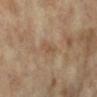follow-up = total-body-photography surveillance lesion; no biopsy
imaging modality = ~15 mm tile from a whole-body skin photo
patient = female, in their mid- to late 70s
diameter = about 2.5 mm
illumination = cross-polarized
location = the right lower leg